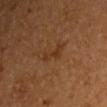patient: male, in their mid- to late 50s
imaging modality: ~15 mm tile from a whole-body skin photo
body site: the left upper arm
lighting: cross-polarized illumination